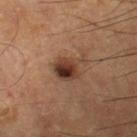biopsy_status: not biopsied; imaged during a skin examination
site: leg
patient:
  sex: male
  age_approx: 55
image:
  source: total-body photography crop
  field_of_view_mm: 15